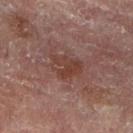subject: female, about 80 years old | illumination: cross-polarized illumination | image source: total-body-photography crop, ~15 mm field of view | location: the right leg.